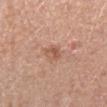Part of a total-body skin-imaging series; this lesion was reviewed on a skin check and was not flagged for biopsy.
The total-body-photography lesion software estimated an eccentricity of roughly 0.75 and two-axis asymmetry of about 0.2. And it measured border irregularity of about 1.5 on a 0–10 scale and a peripheral color-asymmetry measure near 2. It also reported a classifier nevus-likeness of about 5/100 and lesion-presence confidence of about 100/100.
This image is a 15 mm lesion crop taken from a total-body photograph.
The patient is a female about 50 years old.
On the right lower leg.
Imaged with white-light lighting.
Approximately 3 mm at its widest.